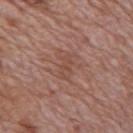No biopsy was performed on this lesion — it was imaged during a full skin examination and was not determined to be concerning. From the back. A male subject, roughly 70 years of age. A 15 mm close-up tile from a total-body photography series done for melanoma screening. Imaged with white-light lighting. Measured at roughly 3 mm in maximum diameter.